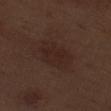- workup · imaged on a skin check; not biopsied
- anatomic site · the right thigh
- patient · male, aged around 70
- imaging modality · ~15 mm tile from a whole-body skin photo